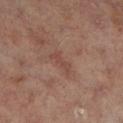Clinical impression:
Recorded during total-body skin imaging; not selected for excision or biopsy.
Image and clinical context:
The subject is a male approximately 65 years of age. Approximately 3 mm at its widest. Located on the right lower leg. A 15 mm crop from a total-body photograph taken for skin-cancer surveillance.